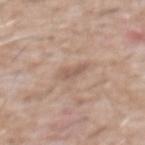{
  "biopsy_status": "not biopsied; imaged during a skin examination"
}